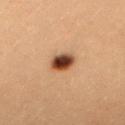Acquisition and patient details: The lesion is on the head or neck. A 15 mm close-up tile from a total-body photography series done for melanoma screening. About 2.5 mm across. The patient is a female in their 20s.